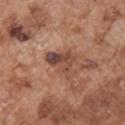notes: no biopsy performed (imaged during a skin exam)
subject: male, in their mid-70s
anatomic site: the right upper arm
imaging modality: ~15 mm tile from a whole-body skin photo